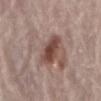Q: Is there a histopathology result?
A: imaged on a skin check; not biopsied
Q: Who is the patient?
A: female, aged 63 to 67
Q: What is the imaging modality?
A: total-body-photography crop, ~15 mm field of view
Q: What is the anatomic site?
A: the abdomen
Q: How large is the lesion?
A: about 7.5 mm
Q: Illumination type?
A: white-light